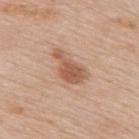A male patient roughly 80 years of age. Automated image analysis of the tile measured a footprint of about 7.5 mm². And it measured a mean CIELAB color near L≈56 a*≈22 b*≈32, roughly 11 lightness units darker than nearby skin, and a normalized lesion–skin contrast near 8. A 15 mm crop from a total-body photograph taken for skin-cancer surveillance. The lesion is located on the upper back. The recorded lesion diameter is about 4.5 mm. The tile uses white-light illumination.